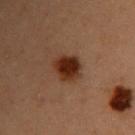subject: female, in their 40s
anatomic site: the upper back
lesion diameter: ~3.5 mm (longest diameter)
imaging modality: ~15 mm tile from a whole-body skin photo